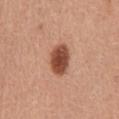notes = catalogued during a skin exam; not biopsied
anatomic site = the mid back
imaging modality = total-body-photography crop, ~15 mm field of view
subject = male, aged 43 to 47